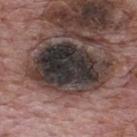Background:
A lesion tile, about 15 mm wide, cut from a 3D total-body photograph. The subject is a male aged around 70. On the upper back.